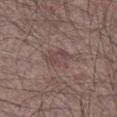biopsy status: catalogued during a skin exam; not biopsied | tile lighting: white-light | image source: 15 mm crop, total-body photography | body site: the right lower leg | patient: male, aged 63 to 67 | lesion diameter: ~3.5 mm (longest diameter).